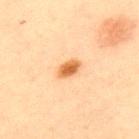The lesion was photographed on a routine skin check and not biopsied; there is no pathology result.
This is a cross-polarized tile.
The subject is a male roughly 60 years of age.
Longest diameter approximately 3 mm.
The total-body-photography lesion software estimated a lesion-to-skin contrast of about 9.5 (normalized; higher = more distinct).
The lesion is on the upper back.
Cropped from a whole-body photographic skin survey; the tile spans about 15 mm.